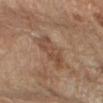notes — no biopsy performed (imaged during a skin exam); tile lighting — cross-polarized illumination; automated lesion analysis — a shape eccentricity near 0.75 and a symmetry-axis asymmetry near 0.25; body site — the right forearm; image — ~15 mm crop, total-body skin-cancer survey; subject — female, roughly 60 years of age; lesion size — ≈4.5 mm.